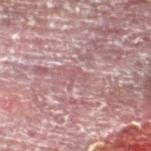notes: no biopsy performed (imaged during a skin exam)
lesion diameter: ~2.5 mm (longest diameter)
subject: male, approximately 55 years of age
illumination: cross-polarized illumination
image source: ~15 mm tile from a whole-body skin photo
automated metrics: an area of roughly 2.5 mm², an outline eccentricity of about 0.9 (0 = round, 1 = elongated), and a shape-asymmetry score of about 0.4 (0 = symmetric); an automated nevus-likeness rating near 0 out of 100
location: the left lower leg